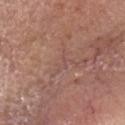The lesion is on the head or neck.
An algorithmic analysis of the crop reported a classifier nevus-likeness of about 0/100.
This is a white-light tile.
About 3 mm across.
The patient is a female aged approximately 65.
A lesion tile, about 15 mm wide, cut from a 3D total-body photograph.
The biopsy diagnosis was a nodular basal cell carcinoma, classified as a skin cancer.